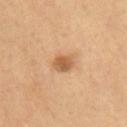  biopsy_status: not biopsied; imaged during a skin examination
  image:
    source: total-body photography crop
    field_of_view_mm: 15
  lesion_size:
    long_diameter_mm_approx: 2.5
  patient:
    sex: male
    age_approx: 70
  site: front of the torso